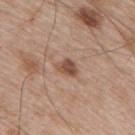Clinical impression:
Recorded during total-body skin imaging; not selected for excision or biopsy.
Clinical summary:
A male patient about 65 years old. From the right upper arm. This is a white-light tile. Measured at roughly 3 mm in maximum diameter. Cropped from a whole-body photographic skin survey; the tile spans about 15 mm.